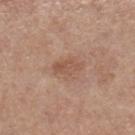  image:
    source: total-body photography crop
    field_of_view_mm: 15
  patient:
    sex: female
    age_approx: 45
  lesion_size:
    long_diameter_mm_approx: 3.5
  lighting: white-light
  automated_metrics:
    area_mm2_approx: 4.0
    eccentricity: 0.85
    shape_asymmetry: 0.2
    border_irregularity_0_10: 2.5
    color_variation_0_10: 2.0
    peripheral_color_asymmetry: 0.5
  site: right lower leg